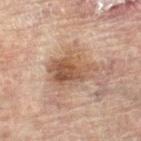Clinical impression:
The lesion was tiled from a total-body skin photograph and was not biopsied.
Acquisition and patient details:
The subject is a female aged 78 to 82. From the left leg. A lesion tile, about 15 mm wide, cut from a 3D total-body photograph. Imaged with cross-polarized lighting.